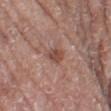Impression: Recorded during total-body skin imaging; not selected for excision or biopsy. Background: A female subject, in their mid- to late 70s. Imaged with white-light lighting. The total-body-photography lesion software estimated a within-lesion color-variation index near 3/10 and peripheral color asymmetry of about 1. The lesion's longest dimension is about 3 mm. A lesion tile, about 15 mm wide, cut from a 3D total-body photograph. From the left thigh.